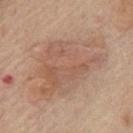| field | value |
|---|---|
| follow-up | imaged on a skin check; not biopsied |
| anatomic site | the chest |
| image | 15 mm crop, total-body photography |
| subject | male, aged approximately 75 |